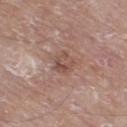Notes:
• patient: male, aged 78–82
• TBP lesion metrics: a shape eccentricity near 0.65; a within-lesion color-variation index near 5.5/10 and radial color variation of about 2; an automated nevus-likeness rating near 0 out of 100 and lesion-presence confidence of about 100/100
• acquisition: total-body-photography crop, ~15 mm field of view
• illumination: white-light illumination
• lesion size: about 3 mm
• location: the right thigh